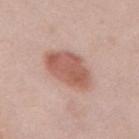notes — catalogued during a skin exam; not biopsied | automated lesion analysis — a footprint of about 17 mm², an eccentricity of roughly 0.85, and two-axis asymmetry of about 0.15 | lesion diameter — ≈7 mm | subject — female, about 65 years old | tile lighting — white-light | imaging modality — total-body-photography crop, ~15 mm field of view | body site — the mid back.